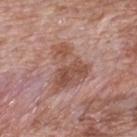Assessment:
Part of a total-body skin-imaging series; this lesion was reviewed on a skin check and was not flagged for biopsy.
Context:
Measured at roughly 5.5 mm in maximum diameter. A region of skin cropped from a whole-body photographic capture, roughly 15 mm wide. The total-body-photography lesion software estimated about 9 CIELAB-L* units darker than the surrounding skin and a lesion-to-skin contrast of about 7 (normalized; higher = more distinct). This is a white-light tile. The patient is a male aged around 70. The lesion is located on the mid back.